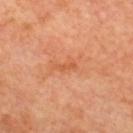Clinical impression:
Part of a total-body skin-imaging series; this lesion was reviewed on a skin check and was not flagged for biopsy.
Clinical summary:
The lesion's longest dimension is about 3 mm. The patient is a male approximately 60 years of age. Automated image analysis of the tile measured an area of roughly 2.5 mm² and two-axis asymmetry of about 0.5. It also reported a border-irregularity index near 5/10, internal color variation of about 0 on a 0–10 scale, and peripheral color asymmetry of about 0. And it measured lesion-presence confidence of about 100/100. A 15 mm close-up extracted from a 3D total-body photography capture. Imaged with cross-polarized lighting. On the upper back.